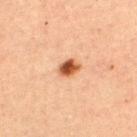Assessment: No biopsy was performed on this lesion — it was imaged during a full skin examination and was not determined to be concerning. Background: The lesion is on the back. Approximately 2.5 mm at its widest. A male subject, about 30 years old. A 15 mm close-up tile from a total-body photography series done for melanoma screening. This is a cross-polarized tile.